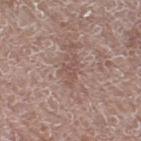No biopsy was performed on this lesion — it was imaged during a full skin examination and was not determined to be concerning. This image is a 15 mm lesion crop taken from a total-body photograph. A female subject, approximately 65 years of age. This is a white-light tile. Located on the right thigh.